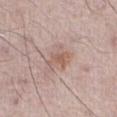{
  "biopsy_status": "not biopsied; imaged during a skin examination",
  "image": {
    "source": "total-body photography crop",
    "field_of_view_mm": 15
  },
  "lesion_size": {
    "long_diameter_mm_approx": 3.5
  },
  "automated_metrics": {
    "area_mm2_approx": 6.5,
    "eccentricity": 0.65,
    "shape_asymmetry": 0.4,
    "color_variation_0_10": 3.0,
    "peripheral_color_asymmetry": 1.0
  },
  "lighting": "white-light",
  "patient": {
    "sex": "male",
    "age_approx": 65
  },
  "site": "abdomen"
}